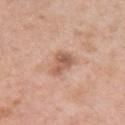Imaged during a routine full-body skin examination; the lesion was not biopsied and no histopathology is available.
A female subject, roughly 40 years of age.
The recorded lesion diameter is about 3 mm.
Located on the right upper arm.
A lesion tile, about 15 mm wide, cut from a 3D total-body photograph.
Captured under white-light illumination.